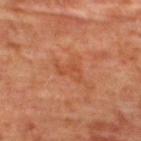Q: Is there a histopathology result?
A: catalogued during a skin exam; not biopsied
Q: Where on the body is the lesion?
A: the upper back
Q: What is the imaging modality?
A: ~15 mm crop, total-body skin-cancer survey
Q: Who is the patient?
A: female, in their mid- to late 40s
Q: How large is the lesion?
A: ~3.5 mm (longest diameter)